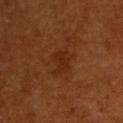Captured during whole-body skin photography for melanoma surveillance; the lesion was not biopsied.
This image is a 15 mm lesion crop taken from a total-body photograph.
Located on the upper back.
About 2.5 mm across.
A male patient aged around 60.
This is a cross-polarized tile.